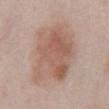Case summary:
– notes · catalogued during a skin exam; not biopsied
– patient · male, aged 53–57
– image source · ~15 mm crop, total-body skin-cancer survey
– lesion diameter · about 7.5 mm
– lighting · white-light illumination
– site · the chest